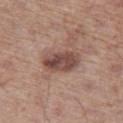Findings:
– workup: imaged on a skin check; not biopsied
– illumination: white-light illumination
– location: the left thigh
– imaging modality: total-body-photography crop, ~15 mm field of view
– subject: male, approximately 65 years of age
– size: ≈4.5 mm
– automated lesion analysis: a footprint of about 10 mm², a shape eccentricity near 0.8, and a shape-asymmetry score of about 0.15 (0 = symmetric); a border-irregularity index near 1.5/10 and a peripheral color-asymmetry measure near 2